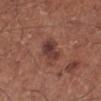Impression:
No biopsy was performed on this lesion — it was imaged during a full skin examination and was not determined to be concerning.
Context:
Automated tile analysis of the lesion measured a lesion area of about 6.5 mm², a shape eccentricity near 0.7, and a shape-asymmetry score of about 0.25 (0 = symmetric). And it measured a border-irregularity index near 2.5/10, a within-lesion color-variation index near 5/10, and a peripheral color-asymmetry measure near 2. The analysis additionally found a detector confidence of about 100 out of 100 that the crop contains a lesion. On the left lower leg. The patient is a male about 70 years old. This is a white-light tile. The recorded lesion diameter is about 3.5 mm. A 15 mm close-up extracted from a 3D total-body photography capture.